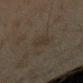biopsy_status: not biopsied; imaged during a skin examination
patient:
  sex: male
  age_approx: 45
image:
  source: total-body photography crop
  field_of_view_mm: 15
lesion_size:
  long_diameter_mm_approx: 3.0
lighting: cross-polarized
site: arm